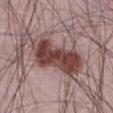follow-up=catalogued during a skin exam; not biopsied | site=the front of the torso | tile lighting=white-light illumination | size=~8 mm (longest diameter) | image=15 mm crop, total-body photography | patient=male, about 65 years old | automated metrics=an area of roughly 25 mm² and a shape eccentricity near 0.85; a mean CIELAB color near L≈44 a*≈21 b*≈21, a lesion–skin lightness drop of about 15, and a normalized border contrast of about 11.